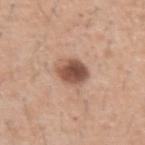Assessment:
Recorded during total-body skin imaging; not selected for excision or biopsy.
Image and clinical context:
A roughly 15 mm field-of-view crop from a total-body skin photograph. The subject is a male roughly 40 years of age. The total-body-photography lesion software estimated a classifier nevus-likeness of about 75/100. On the left upper arm.